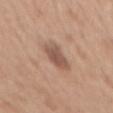Impression:
The lesion was photographed on a routine skin check and not biopsied; there is no pathology result.
Acquisition and patient details:
Located on the mid back. Captured under white-light illumination. Measured at roughly 4.5 mm in maximum diameter. Cropped from a total-body skin-imaging series; the visible field is about 15 mm. Automated tile analysis of the lesion measured a mean CIELAB color near L≈53 a*≈19 b*≈27, a lesion–skin lightness drop of about 11, and a normalized lesion–skin contrast near 8. The analysis additionally found internal color variation of about 2.5 on a 0–10 scale and a peripheral color-asymmetry measure near 1. It also reported an automated nevus-likeness rating near 85 out of 100 and a lesion-detection confidence of about 100/100. A female patient about 40 years old.